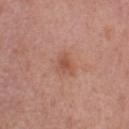- follow-up · no biopsy performed (imaged during a skin exam)
- location · the left upper arm
- patient · male, approximately 55 years of age
- image · 15 mm crop, total-body photography
- illumination · white-light